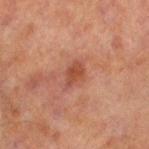biopsy_status: not biopsied; imaged during a skin examination
patient:
  sex: male
  age_approx: 70
image:
  source: total-body photography crop
  field_of_view_mm: 15
site: left lower leg